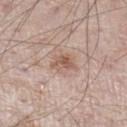Impression: The lesion was photographed on a routine skin check and not biopsied; there is no pathology result. Image and clinical context: The lesion-visualizer software estimated a border-irregularity rating of about 4/10, internal color variation of about 4.5 on a 0–10 scale, and peripheral color asymmetry of about 1.5. The software also gave a nevus-likeness score of about 20/100. Located on the left lower leg. The lesion's longest dimension is about 3 mm. A male patient, aged 58 to 62. Cropped from a total-body skin-imaging series; the visible field is about 15 mm.